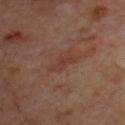Recorded during total-body skin imaging; not selected for excision or biopsy.
This is a cross-polarized tile.
A close-up tile cropped from a whole-body skin photograph, about 15 mm across.
An algorithmic analysis of the crop reported an area of roughly 4.5 mm², a shape eccentricity near 0.9, and two-axis asymmetry of about 0.4. The software also gave a within-lesion color-variation index near 1/10 and radial color variation of about 0.
From the front of the torso.
A male patient, in their 70s.